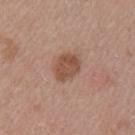follow-up = imaged on a skin check; not biopsied
tile lighting = white-light
body site = the left upper arm
imaging modality = total-body-photography crop, ~15 mm field of view
patient = female, about 45 years old
diameter = about 3.5 mm